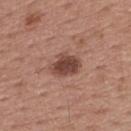location = the upper back | subject = male, aged around 55 | lighting = white-light | acquisition = ~15 mm tile from a whole-body skin photo | size = ≈3.5 mm.